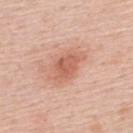workup — no biopsy performed (imaged during a skin exam); subject — male, in their mid-50s; acquisition — 15 mm crop, total-body photography; site — the upper back; lesion diameter — ~3.5 mm (longest diameter); tile lighting — white-light illumination.